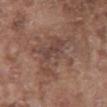<record>
<biopsy_status>not biopsied; imaged during a skin examination</biopsy_status>
<image>
  <source>total-body photography crop</source>
  <field_of_view_mm>15</field_of_view_mm>
</image>
<site>chest</site>
<lesion_size>
  <long_diameter_mm_approx>8.0</long_diameter_mm_approx>
</lesion_size>
<automated_metrics>
  <cielab_L>45</cielab_L>
  <cielab_a>18</cielab_a>
  <cielab_b>24</cielab_b>
  <vs_skin_darker_L>7.0</vs_skin_darker_L>
  <vs_skin_contrast_norm>6.0</vs_skin_contrast_norm>
  <border_irregularity_0_10>8.5</border_irregularity_0_10>
  <color_variation_0_10>4.5</color_variation_0_10>
  <peripheral_color_asymmetry>1.5</peripheral_color_asymmetry>
  <nevus_likeness_0_100>0</nevus_likeness_0_100>
  <lesion_detection_confidence_0_100>80</lesion_detection_confidence_0_100>
</automated_metrics>
<lighting>white-light</lighting>
<patient>
  <sex>male</sex>
  <age_approx>75</age_approx>
</patient>
</record>